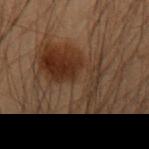{"site": "right forearm", "lighting": "cross-polarized", "patient": {"sex": "male", "age_approx": 55}, "image": {"source": "total-body photography crop", "field_of_view_mm": 15}}